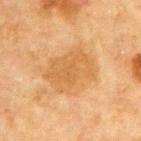  biopsy_status: not biopsied; imaged during a skin examination
  patient:
    sex: male
    age_approx: 65
  automated_metrics:
    area_mm2_approx: 17.0
    eccentricity: 0.75
    shape_asymmetry: 0.2
    cielab_L: 50
    cielab_a: 18
    cielab_b: 37
    vs_skin_darker_L: 7.0
    vs_skin_contrast_norm: 6.0
    nevus_likeness_0_100: 5
    lesion_detection_confidence_0_100: 100
  lighting: cross-polarized
  image:
    source: total-body photography crop
    field_of_view_mm: 15
  site: chest